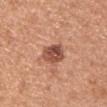Q: Was this lesion biopsied?
A: catalogued during a skin exam; not biopsied
Q: Where on the body is the lesion?
A: the left upper arm
Q: What are the patient's age and sex?
A: female, aged 48–52
Q: How was this image acquired?
A: 15 mm crop, total-body photography